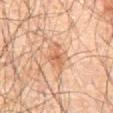biopsy_status: not biopsied; imaged during a skin examination
patient:
  sex: male
  age_approx: 60
lesion_size:
  long_diameter_mm_approx: 3.0
automated_metrics:
  cielab_L: 48
  cielab_a: 19
  cielab_b: 28
  vs_skin_darker_L: 7.0
  color_variation_0_10: 2.5
  peripheral_color_asymmetry: 1.0
  nevus_likeness_0_100: 0
  lesion_detection_confidence_0_100: 100
image:
  source: total-body photography crop
  field_of_view_mm: 15
site: abdomen
lighting: cross-polarized